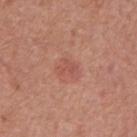Impression: No biopsy was performed on this lesion — it was imaged during a full skin examination and was not determined to be concerning. Image and clinical context: A male patient, in their mid- to late 60s. A 15 mm close-up tile from a total-body photography series done for melanoma screening. The lesion-visualizer software estimated a lesion–skin lightness drop of about 7 and a lesion-to-skin contrast of about 4.5 (normalized; higher = more distinct). It also reported border irregularity of about 3.5 on a 0–10 scale and a within-lesion color-variation index near 1.5/10. On the back. The recorded lesion diameter is about 2.5 mm.